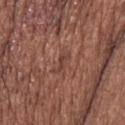Notes:
* follow-up — catalogued during a skin exam; not biopsied
* image-analysis metrics — about 7 CIELAB-L* units darker than the surrounding skin and a normalized border contrast of about 5.5
* image source — 15 mm crop, total-body photography
* diameter — ≈3 mm
* location — the upper back
* subject — male, roughly 75 years of age